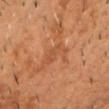Clinical impression:
Part of a total-body skin-imaging series; this lesion was reviewed on a skin check and was not flagged for biopsy.
Acquisition and patient details:
A male subject, roughly 50 years of age. The lesion-visualizer software estimated border irregularity of about 6.5 on a 0–10 scale, a within-lesion color-variation index near 2.5/10, and a peripheral color-asymmetry measure near 0.5. It also reported a classifier nevus-likeness of about 0/100 and a lesion-detection confidence of about 95/100. On the chest. A 15 mm crop from a total-body photograph taken for skin-cancer surveillance.